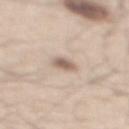Imaged during a routine full-body skin examination; the lesion was not biopsied and no histopathology is available. A male patient aged around 60. A 15 mm crop from a total-body photograph taken for skin-cancer surveillance. The lesion's longest dimension is about 2.5 mm. Automated image analysis of the tile measured a lesion area of about 3.5 mm², an eccentricity of roughly 0.7, and a symmetry-axis asymmetry near 0.25. It also reported an average lesion color of about L≈61 a*≈15 b*≈26 (CIELAB) and about 13 CIELAB-L* units darker than the surrounding skin. The lesion is located on the mid back. This is a white-light tile.